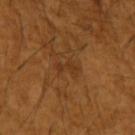Q: Is there a histopathology result?
A: imaged on a skin check; not biopsied
Q: Where on the body is the lesion?
A: the left upper arm
Q: How was this image acquired?
A: ~15 mm crop, total-body skin-cancer survey
Q: Who is the patient?
A: male, aged approximately 65
Q: How large is the lesion?
A: ≈2.5 mm